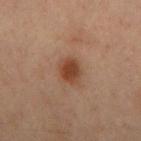biopsy status: imaged on a skin check; not biopsied | image source: ~15 mm crop, total-body skin-cancer survey | lighting: cross-polarized | automated lesion analysis: a lesion area of about 5 mm², a shape eccentricity near 0.7, and two-axis asymmetry of about 0.2; an average lesion color of about L≈31 a*≈18 b*≈25 (CIELAB) and roughly 9 lightness units darker than nearby skin; a lesion-detection confidence of about 100/100 | site: the mid back | subject: male, approximately 60 years of age | size: ~3 mm (longest diameter).